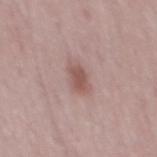This lesion was catalogued during total-body skin photography and was not selected for biopsy.
Automated tile analysis of the lesion measured a border-irregularity index near 2.5/10, a color-variation rating of about 3/10, and a peripheral color-asymmetry measure near 1. And it measured an automated nevus-likeness rating near 60 out of 100 and a detector confidence of about 100 out of 100 that the crop contains a lesion.
The subject is a male about 50 years old.
A 15 mm close-up extracted from a 3D total-body photography capture.
Captured under white-light illumination.
Approximately 3 mm at its widest.
On the mid back.